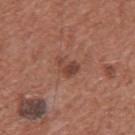Clinical impression:
Imaged during a routine full-body skin examination; the lesion was not biopsied and no histopathology is available.
Context:
A male subject, aged 43–47. From the chest. A 15 mm close-up extracted from a 3D total-body photography capture.